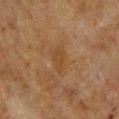Notes:
- notes: imaged on a skin check; not biopsied
- site: the front of the torso
- acquisition: ~15 mm tile from a whole-body skin photo
- diameter: about 3 mm
- subject: female, aged 53–57
- TBP lesion metrics: an outline eccentricity of about 0.9 (0 = round, 1 = elongated); an average lesion color of about L≈36 a*≈17 b*≈31 (CIELAB), about 5 CIELAB-L* units darker than the surrounding skin, and a normalized lesion–skin contrast near 5; a nevus-likeness score of about 0/100 and a detector confidence of about 100 out of 100 that the crop contains a lesion
- tile lighting: cross-polarized